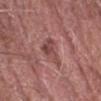follow-up = no biopsy performed (imaged during a skin exam) | acquisition = 15 mm crop, total-body photography | location = the right forearm | lighting = white-light illumination | subject = male, about 80 years old.